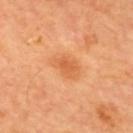Context:
A male patient, approximately 65 years of age. Longest diameter approximately 4 mm. The lesion is located on the back. A 15 mm close-up tile from a total-body photography series done for melanoma screening. The lesion-visualizer software estimated a lesion area of about 7 mm², a shape eccentricity near 0.75, and a shape-asymmetry score of about 0.25 (0 = symmetric). The software also gave an average lesion color of about L≈62 a*≈29 b*≈44 (CIELAB), roughly 8 lightness units darker than nearby skin, and a normalized lesion–skin contrast near 5.5.